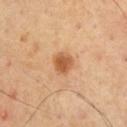Case summary:
- biopsy status · catalogued during a skin exam; not biopsied
- body site · the chest
- TBP lesion metrics · a lesion area of about 5.5 mm², a shape eccentricity near 0.45, and a symmetry-axis asymmetry near 0.25; roughly 12 lightness units darker than nearby skin and a normalized lesion–skin contrast near 8.5
- subject · male, aged approximately 70
- illumination · cross-polarized
- image source · total-body-photography crop, ~15 mm field of view